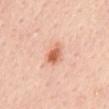The subject is a female aged 43 to 47. A roughly 15 mm field-of-view crop from a total-body skin photograph. The lesion's longest dimension is about 3 mm. On the mid back.